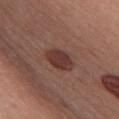Impression: Captured during whole-body skin photography for melanoma surveillance; the lesion was not biopsied. Image and clinical context: The tile uses white-light illumination. The total-body-photography lesion software estimated a footprint of about 7.5 mm², a shape eccentricity near 0.8, and two-axis asymmetry of about 0.2. And it measured a mean CIELAB color near L≈36 a*≈22 b*≈23 and about 10 CIELAB-L* units darker than the surrounding skin. Located on the chest. A female patient aged 38 to 42. A region of skin cropped from a whole-body photographic capture, roughly 15 mm wide. Measured at roughly 4 mm in maximum diameter.